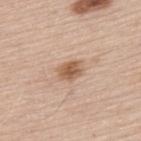Imaged during a routine full-body skin examination; the lesion was not biopsied and no histopathology is available. A lesion tile, about 15 mm wide, cut from a 3D total-body photograph. A male subject roughly 65 years of age. The lesion-visualizer software estimated a lesion area of about 5.5 mm² and two-axis asymmetry of about 0.25. And it measured a mean CIELAB color near L≈58 a*≈19 b*≈32, roughly 12 lightness units darker than nearby skin, and a normalized border contrast of about 8. The software also gave border irregularity of about 2 on a 0–10 scale, a within-lesion color-variation index near 3.5/10, and radial color variation of about 1. Located on the upper back.